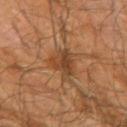Assessment:
Recorded during total-body skin imaging; not selected for excision or biopsy.
Background:
A male patient, in their mid-60s. Approximately 4.5 mm at its widest. This image is a 15 mm lesion crop taken from a total-body photograph. Automated image analysis of the tile measured a nevus-likeness score of about 35/100 and a detector confidence of about 100 out of 100 that the crop contains a lesion. This is a cross-polarized tile. The lesion is on the arm.